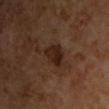Assessment: The lesion was photographed on a routine skin check and not biopsied; there is no pathology result. Acquisition and patient details: Cropped from a total-body skin-imaging series; the visible field is about 15 mm. Imaged with cross-polarized lighting. About 3.5 mm across. An algorithmic analysis of the crop reported a lesion area of about 6 mm², an eccentricity of roughly 0.8, and two-axis asymmetry of about 0.3. The analysis additionally found a lesion-to-skin contrast of about 10 (normalized; higher = more distinct). The software also gave a border-irregularity rating of about 3/10 and a color-variation rating of about 3.5/10. A male patient aged 63 to 67. The lesion is located on the chest.